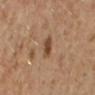This lesion was catalogued during total-body skin photography and was not selected for biopsy. From the arm. The patient is a female aged approximately 40. A 15 mm crop from a total-body photograph taken for skin-cancer surveillance. Approximately 2.5 mm at its widest.